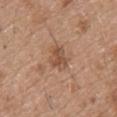biopsy status = total-body-photography surveillance lesion; no biopsy | acquisition = 15 mm crop, total-body photography | subject = male, aged 48 to 52 | location = the upper back.